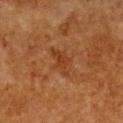No biopsy was performed on this lesion — it was imaged during a full skin examination and was not determined to be concerning.
The total-body-photography lesion software estimated a footprint of about 6 mm² and two-axis asymmetry of about 0.35. The software also gave a border-irregularity rating of about 4.5/10 and internal color variation of about 2 on a 0–10 scale. The analysis additionally found a lesion-detection confidence of about 100/100.
Longest diameter approximately 3.5 mm.
A female patient in their 50s.
A 15 mm close-up tile from a total-body photography series done for melanoma screening.
The lesion is located on the chest.
The tile uses cross-polarized illumination.